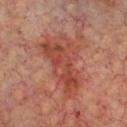Imaged during a routine full-body skin examination; the lesion was not biopsied and no histopathology is available. Located on the chest. This image is a 15 mm lesion crop taken from a total-body photograph. The patient is approximately 65 years of age.